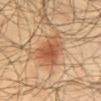site: mid back
image:
  source: total-body photography crop
  field_of_view_mm: 15
patient:
  sex: male
  age_approx: 50